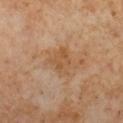The lesion was photographed on a routine skin check and not biopsied; there is no pathology result. A region of skin cropped from a whole-body photographic capture, roughly 15 mm wide. About 3 mm across. A female patient, aged approximately 50. This is a cross-polarized tile. From the right lower leg.